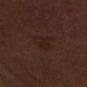histopathologic diagnosis: a squamous cell carcinoma in situ (malignant).